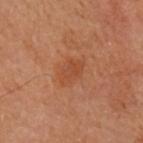Recorded during total-body skin imaging; not selected for excision or biopsy. From the right arm. The patient is a female in their 60s. The lesion's longest dimension is about 4 mm. The tile uses cross-polarized illumination. This image is a 15 mm lesion crop taken from a total-body photograph. The total-body-photography lesion software estimated an average lesion color of about L≈41 a*≈23 b*≈32 (CIELAB) and a lesion–skin lightness drop of about 6.